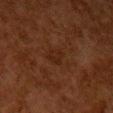Assessment: Captured during whole-body skin photography for melanoma surveillance; the lesion was not biopsied. Acquisition and patient details: From the left upper arm. A female subject aged 48–52. A 15 mm close-up extracted from a 3D total-body photography capture.